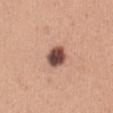Impression:
Imaged during a routine full-body skin examination; the lesion was not biopsied and no histopathology is available.
Acquisition and patient details:
The total-body-photography lesion software estimated roughly 20 lightness units darker than nearby skin and a normalized lesion–skin contrast near 13.5. The analysis additionally found a border-irregularity rating of about 2/10, a within-lesion color-variation index near 5.5/10, and peripheral color asymmetry of about 1.5. The subject is a female about 30 years old. Approximately 3 mm at its widest. From the abdomen. Cropped from a whole-body photographic skin survey; the tile spans about 15 mm.